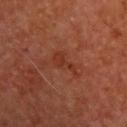Clinical summary:
Located on the upper back. A male subject, in their mid-60s. A lesion tile, about 15 mm wide, cut from a 3D total-body photograph. Measured at roughly 4 mm in maximum diameter.